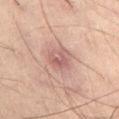size = ~3 mm (longest diameter)
tile lighting = cross-polarized
TBP lesion metrics = a footprint of about 7 mm², an eccentricity of roughly 0.4, and two-axis asymmetry of about 0.2; a detector confidence of about 100 out of 100 that the crop contains a lesion
site = the abdomen
patient = male, aged approximately 60
imaging modality = ~15 mm crop, total-body skin-cancer survey